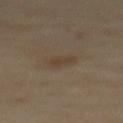workup = total-body-photography surveillance lesion; no biopsy
image-analysis metrics = a lesion color around L≈39 a*≈11 b*≈25 in CIELAB, about 5 CIELAB-L* units darker than the surrounding skin, and a normalized lesion–skin contrast near 5; an automated nevus-likeness rating near 0 out of 100 and lesion-presence confidence of about 100/100
lesion diameter = about 3 mm
tile lighting = cross-polarized illumination
subject = female, aged 58 to 62
image = total-body-photography crop, ~15 mm field of view
site = the upper back